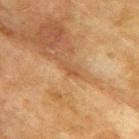The lesion was tiled from a total-body skin photograph and was not biopsied.
The lesion is located on the upper back.
Longest diameter approximately 2.5 mm.
This is a cross-polarized tile.
A close-up tile cropped from a whole-body skin photograph, about 15 mm across.
A male subject aged 73–77.